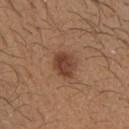Q: Was this lesion biopsied?
A: imaged on a skin check; not biopsied
Q: How large is the lesion?
A: about 4 mm
Q: Where on the body is the lesion?
A: the upper back
Q: What lighting was used for the tile?
A: white-light
Q: Automated lesion metrics?
A: a lesion area of about 7.5 mm², a shape eccentricity near 0.7, and two-axis asymmetry of about 0.15; an average lesion color of about L≈42 a*≈21 b*≈30 (CIELAB) and about 11 CIELAB-L* units darker than the surrounding skin; a border-irregularity rating of about 1.5/10 and radial color variation of about 1; an automated nevus-likeness rating near 95 out of 100 and a detector confidence of about 100 out of 100 that the crop contains a lesion
Q: What is the imaging modality?
A: ~15 mm tile from a whole-body skin photo
Q: Who is the patient?
A: male, roughly 30 years of age